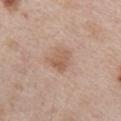biopsy status: imaged on a skin check; not biopsied
patient: male, about 65 years old
image source: 15 mm crop, total-body photography
anatomic site: the front of the torso